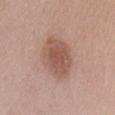{"site": "chest", "lighting": "white-light", "patient": {"sex": "female", "age_approx": 60}, "image": {"source": "total-body photography crop", "field_of_view_mm": 15}}